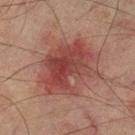Part of a total-body skin-imaging series; this lesion was reviewed on a skin check and was not flagged for biopsy.
Approximately 7 mm at its widest.
Automated image analysis of the tile measured roughly 9 lightness units darker than nearby skin. The software also gave a color-variation rating of about 5.5/10 and radial color variation of about 2.
A roughly 15 mm field-of-view crop from a total-body skin photograph.
Located on the left lower leg.
A male subject in their mid-70s.